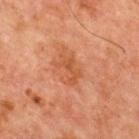Assessment: Captured during whole-body skin photography for melanoma surveillance; the lesion was not biopsied. Background: A close-up tile cropped from a whole-body skin photograph, about 15 mm across. The patient is a male roughly 65 years of age. The tile uses cross-polarized illumination. About 3.5 mm across. The lesion is on the front of the torso.